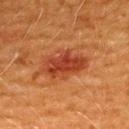notes = no biopsy performed (imaged during a skin exam) | site = the back | diameter = ≈5.5 mm | subject = female, roughly 40 years of age | image-analysis metrics = a lesion–skin lightness drop of about 10 | tile lighting = cross-polarized | acquisition = ~15 mm crop, total-body skin-cancer survey.